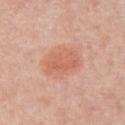No biopsy was performed on this lesion — it was imaged during a full skin examination and was not determined to be concerning. Longest diameter approximately 4.5 mm. The patient is a female in their 60s. Located on the chest. The tile uses white-light illumination. Automated image analysis of the tile measured an area of roughly 10 mm², an eccentricity of roughly 0.75, and two-axis asymmetry of about 0.25. The software also gave an automated nevus-likeness rating near 85 out of 100 and lesion-presence confidence of about 100/100. A 15 mm close-up tile from a total-body photography series done for melanoma screening.